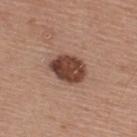Impression: Captured during whole-body skin photography for melanoma surveillance; the lesion was not biopsied. Acquisition and patient details: Approximately 4.5 mm at its widest. The lesion-visualizer software estimated a color-variation rating of about 4/10 and a peripheral color-asymmetry measure near 1.5. The lesion is on the upper back. Captured under white-light illumination. A male subject about 55 years old. A lesion tile, about 15 mm wide, cut from a 3D total-body photograph.